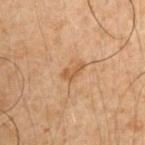Recorded during total-body skin imaging; not selected for excision or biopsy.
This is a cross-polarized tile.
From the right upper arm.
The subject is a male roughly 50 years of age.
Measured at roughly 3 mm in maximum diameter.
A lesion tile, about 15 mm wide, cut from a 3D total-body photograph.